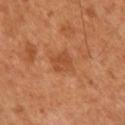Part of a total-body skin-imaging series; this lesion was reviewed on a skin check and was not flagged for biopsy.
The lesion-visualizer software estimated an eccentricity of roughly 0.6. The analysis additionally found roughly 7 lightness units darker than nearby skin and a lesion-to-skin contrast of about 5.5 (normalized; higher = more distinct). And it measured a classifier nevus-likeness of about 5/100 and lesion-presence confidence of about 100/100.
A close-up tile cropped from a whole-body skin photograph, about 15 mm across.
A male patient, in their 50s.
The tile uses cross-polarized illumination.
The lesion is on the right upper arm.